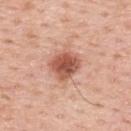notes: no biopsy performed (imaged during a skin exam).